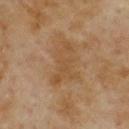| field | value |
|---|---|
| biopsy status | catalogued during a skin exam; not biopsied |
| image source | ~15 mm crop, total-body skin-cancer survey |
| patient | female, aged approximately 60 |
| site | the back |